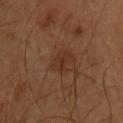Impression:
The lesion was tiled from a total-body skin photograph and was not biopsied.
Image and clinical context:
A male subject about 55 years old. The lesion is located on the chest. A lesion tile, about 15 mm wide, cut from a 3D total-body photograph. Measured at roughly 3 mm in maximum diameter.